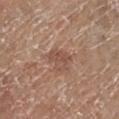{
  "biopsy_status": "not biopsied; imaged during a skin examination",
  "lighting": "white-light",
  "lesion_size": {
    "long_diameter_mm_approx": 3.0
  },
  "patient": {
    "sex": "female",
    "age_approx": 75
  },
  "site": "left lower leg",
  "image": {
    "source": "total-body photography crop",
    "field_of_view_mm": 15
  }
}